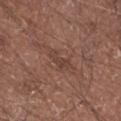The lesion was photographed on a routine skin check and not biopsied; there is no pathology result. Measured at roughly 3 mm in maximum diameter. A 15 mm close-up extracted from a 3D total-body photography capture. The patient is a male aged 63–67. Imaged with white-light lighting. From the right upper arm.